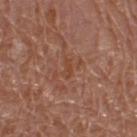* patient: female, approximately 55 years of age
* diameter: ~4 mm (longest diameter)
* acquisition: ~15 mm tile from a whole-body skin photo
* anatomic site: the right thigh